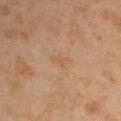{
  "biopsy_status": "not biopsied; imaged during a skin examination",
  "site": "right upper arm",
  "automated_metrics": {
    "shape_asymmetry": 0.35,
    "nevus_likeness_0_100": 0
  },
  "image": {
    "source": "total-body photography crop",
    "field_of_view_mm": 15
  },
  "lesion_size": {
    "long_diameter_mm_approx": 2.5
  },
  "patient": {
    "sex": "male",
    "age_approx": 45
  },
  "lighting": "cross-polarized"
}